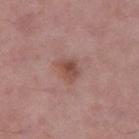biopsy status = no biopsy performed (imaged during a skin exam)
imaging modality = ~15 mm tile from a whole-body skin photo
tile lighting = white-light illumination
subject = male, aged 53–57
site = the left thigh